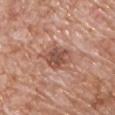Impression: The lesion was photographed on a routine skin check and not biopsied; there is no pathology result. Acquisition and patient details: Measured at roughly 4 mm in maximum diameter. A male subject in their 70s. Imaged with white-light lighting. Located on the chest. Cropped from a whole-body photographic skin survey; the tile spans about 15 mm.